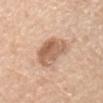The lesion was tiled from a total-body skin photograph and was not biopsied. A 15 mm close-up extracted from a 3D total-body photography capture. A male patient, aged 68–72. Imaged with white-light lighting. From the abdomen. The recorded lesion diameter is about 4.5 mm.